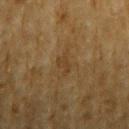Part of a total-body skin-imaging series; this lesion was reviewed on a skin check and was not flagged for biopsy. Captured under cross-polarized illumination. The total-body-photography lesion software estimated an area of roughly 3 mm², an outline eccentricity of about 0.85 (0 = round, 1 = elongated), and a symmetry-axis asymmetry near 0.4. The software also gave a lesion color around L≈34 a*≈13 b*≈30 in CIELAB and a normalized lesion–skin contrast near 5. The analysis additionally found a nevus-likeness score of about 0/100 and a detector confidence of about 95 out of 100 that the crop contains a lesion. The patient is a male aged approximately 85 or older. The lesion's longest dimension is about 2.5 mm. The lesion is located on the right upper arm. A roughly 15 mm field-of-view crop from a total-body skin photograph.